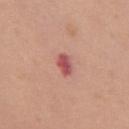Clinical impression: Captured during whole-body skin photography for melanoma surveillance; the lesion was not biopsied. Background: The lesion is on the chest. Cropped from a whole-body photographic skin survey; the tile spans about 15 mm. The subject is a female approximately 50 years of age. Measured at roughly 2.5 mm in maximum diameter.